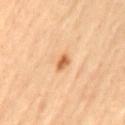workup: total-body-photography surveillance lesion; no biopsy | patient: aged approximately 65 | lesion size: about 2.5 mm | lighting: cross-polarized illumination | automated metrics: an outline eccentricity of about 0.8 (0 = round, 1 = elongated); an average lesion color of about L≈64 a*≈25 b*≈42 (CIELAB), a lesion–skin lightness drop of about 13, and a normalized lesion–skin contrast near 8; a border-irregularity rating of about 2/10 and radial color variation of about 1 | imaging modality: 15 mm crop, total-body photography | location: the lower back.